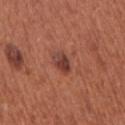  biopsy_status: not biopsied; imaged during a skin examination
  lighting: white-light
  patient:
    sex: female
    age_approx: 65
  lesion_size:
    long_diameter_mm_approx: 3.0
  site: right upper arm
  image:
    source: total-body photography crop
    field_of_view_mm: 15